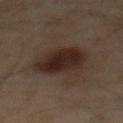Imaged during a routine full-body skin examination; the lesion was not biopsied and no histopathology is available. An algorithmic analysis of the crop reported a lesion area of about 18 mm², an eccentricity of roughly 0.45, and two-axis asymmetry of about 0.15. It also reported about 9 CIELAB-L* units darker than the surrounding skin and a lesion-to-skin contrast of about 10.5 (normalized; higher = more distinct). It also reported a border-irregularity index near 1.5/10, a within-lesion color-variation index near 3.5/10, and a peripheral color-asymmetry measure near 1.5. The software also gave an automated nevus-likeness rating near 95 out of 100. Imaged with cross-polarized lighting. A male subject aged 58 to 62. Longest diameter approximately 5 mm. On the abdomen. A lesion tile, about 15 mm wide, cut from a 3D total-body photograph.